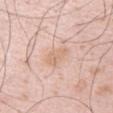<tbp_lesion>
<biopsy_status>not biopsied; imaged during a skin examination</biopsy_status>
<patient>
  <sex>male</sex>
  <age_approx>40</age_approx>
</patient>
<image>
  <source>total-body photography crop</source>
  <field_of_view_mm>15</field_of_view_mm>
</image>
<site>left upper arm</site>
</tbp_lesion>